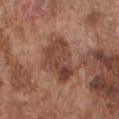<tbp_lesion>
  <biopsy_status>not biopsied; imaged during a skin examination</biopsy_status>
  <image>
    <source>total-body photography crop</source>
    <field_of_view_mm>15</field_of_view_mm>
  </image>
  <lesion_size>
    <long_diameter_mm_approx>6.0</long_diameter_mm_approx>
  </lesion_size>
  <patient>
    <sex>male</sex>
    <age_approx>75</age_approx>
  </patient>
  <site>chest</site>
  <lighting>white-light</lighting>
  <automated_metrics>
    <area_mm2_approx>14.0</area_mm2_approx>
    <eccentricity>0.85</eccentricity>
    <shape_asymmetry>0.3</shape_asymmetry>
    <border_irregularity_0_10>3.5</border_irregularity_0_10>
  </automated_metrics>
</tbp_lesion>